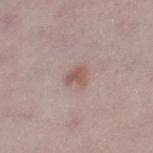| key | value |
|---|---|
| follow-up | total-body-photography surveillance lesion; no biopsy |
| patient | female, approximately 30 years of age |
| diameter | ≈2.5 mm |
| acquisition | ~15 mm crop, total-body skin-cancer survey |
| anatomic site | the left thigh |